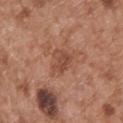{"biopsy_status": "not biopsied; imaged during a skin examination", "image": {"source": "total-body photography crop", "field_of_view_mm": 15}, "patient": {"sex": "male", "age_approx": 55}, "lighting": "white-light", "lesion_size": {"long_diameter_mm_approx": 3.5}, "site": "upper back", "automated_metrics": {"area_mm2_approx": 6.0, "eccentricity": 0.7, "shape_asymmetry": 0.35}}